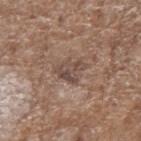Acquisition and patient details: About 3.5 mm across. Automated image analysis of the tile measured a footprint of about 6 mm², a shape eccentricity near 0.75, and a shape-asymmetry score of about 0.45 (0 = symmetric). And it measured a border-irregularity index near 4.5/10 and radial color variation of about 1.5. And it measured an automated nevus-likeness rating near 0 out of 100 and lesion-presence confidence of about 95/100. Cropped from a whole-body photographic skin survey; the tile spans about 15 mm. This is a white-light tile. The patient is a female aged approximately 75. Located on the right forearm.